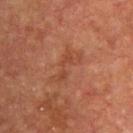A lesion tile, about 15 mm wide, cut from a 3D total-body photograph. On the upper back. The lesion's longest dimension is about 4.5 mm. Captured under cross-polarized illumination. A male subject, in their mid-50s.